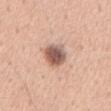This lesion was catalogued during total-body skin photography and was not selected for biopsy.